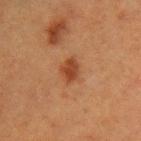<case>
<biopsy_status>not biopsied; imaged during a skin examination</biopsy_status>
<automated_metrics>
  <area_mm2_approx>4.0</area_mm2_approx>
  <eccentricity>0.7</eccentricity>
  <shape_asymmetry>0.25</shape_asymmetry>
  <cielab_L>35</cielab_L>
  <cielab_a>23</cielab_a>
  <cielab_b>31</cielab_b>
  <vs_skin_contrast_norm>8.5</vs_skin_contrast_norm>
  <nevus_likeness_0_100>95</nevus_likeness_0_100>
</automated_metrics>
<lighting>cross-polarized</lighting>
<image>
  <source>total-body photography crop</source>
  <field_of_view_mm>15</field_of_view_mm>
</image>
<lesion_size>
  <long_diameter_mm_approx>2.5</long_diameter_mm_approx>
</lesion_size>
<patient>
  <sex>female</sex>
  <age_approx>40</age_approx>
</patient>
<site>left upper arm</site>
</case>